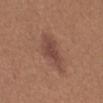| field | value |
|---|---|
| notes | no biopsy performed (imaged during a skin exam) |
| automated lesion analysis | an area of roughly 10 mm², an eccentricity of roughly 0.9, and two-axis asymmetry of about 0.3; a mean CIELAB color near L≈45 a*≈20 b*≈25, about 9 CIELAB-L* units darker than the surrounding skin, and a normalized border contrast of about 7; border irregularity of about 4 on a 0–10 scale, a within-lesion color-variation index near 2.5/10, and a peripheral color-asymmetry measure near 0.5 |
| acquisition | ~15 mm crop, total-body skin-cancer survey |
| diameter | about 5.5 mm |
| illumination | white-light illumination |
| subject | female, aged 23 to 27 |
| location | the back |